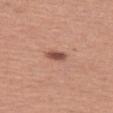Impression:
This lesion was catalogued during total-body skin photography and was not selected for biopsy.
Clinical summary:
The subject is a female aged 48 to 52. The recorded lesion diameter is about 2.5 mm. A 15 mm crop from a total-body photograph taken for skin-cancer surveillance. The lesion-visualizer software estimated an eccentricity of roughly 0.75 and two-axis asymmetry of about 0.25. It also reported roughly 13 lightness units darker than nearby skin. Imaged with white-light lighting. The lesion is on the left thigh.